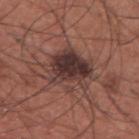Impression: Captured during whole-body skin photography for melanoma surveillance; the lesion was not biopsied. Acquisition and patient details: The tile uses white-light illumination. Longest diameter approximately 6 mm. A male subject, approximately 35 years of age. A region of skin cropped from a whole-body photographic capture, roughly 15 mm wide. Located on the upper back.